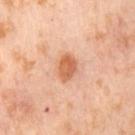This lesion was catalogued during total-body skin photography and was not selected for biopsy.
A close-up tile cropped from a whole-body skin photograph, about 15 mm across.
The tile uses cross-polarized illumination.
The lesion is located on the right thigh.
A female subject, about 55 years old.
Measured at roughly 3.5 mm in maximum diameter.